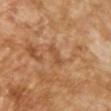This lesion was catalogued during total-body skin photography and was not selected for biopsy. A male subject roughly 65 years of age. Captured under cross-polarized illumination. A 15 mm close-up tile from a total-body photography series done for melanoma screening. Approximately 2.5 mm at its widest.